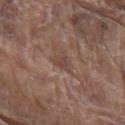patient = male, in their 80s; acquisition = ~15 mm tile from a whole-body skin photo; location = the front of the torso.